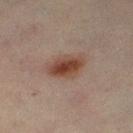Q: Was a biopsy performed?
A: imaged on a skin check; not biopsied
Q: How was this image acquired?
A: ~15 mm crop, total-body skin-cancer survey
Q: Patient demographics?
A: female, aged 43 to 47
Q: Lesion location?
A: the leg